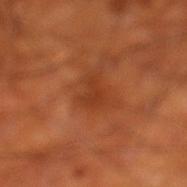{"biopsy_status": "not biopsied; imaged during a skin examination", "image": {"source": "total-body photography crop", "field_of_view_mm": 15}, "lesion_size": {"long_diameter_mm_approx": 3.0}, "lighting": "cross-polarized", "patient": {"sex": "male", "age_approx": 70}, "site": "left lower leg"}